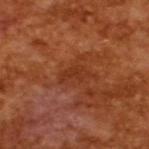Captured during whole-body skin photography for melanoma surveillance; the lesion was not biopsied. A roughly 15 mm field-of-view crop from a total-body skin photograph. Measured at roughly 3.5 mm in maximum diameter. The subject is a male in their mid- to late 60s. Automated tile analysis of the lesion measured an automated nevus-likeness rating near 0 out of 100. The tile uses cross-polarized illumination.